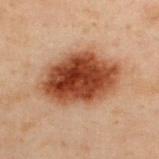biopsy_status: not biopsied; imaged during a skin examination
site: upper back
patient:
  sex: male
  age_approx: 50
lighting: cross-polarized
image:
  source: total-body photography crop
  field_of_view_mm: 15
lesion_size:
  long_diameter_mm_approx: 8.5